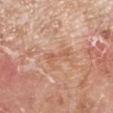No biopsy was performed on this lesion — it was imaged during a full skin examination and was not determined to be concerning. On the left lower leg. A male subject, aged 78–82. Longest diameter approximately 3.5 mm. The lesion-visualizer software estimated a lesion area of about 4 mm², an eccentricity of roughly 0.95, and two-axis asymmetry of about 0.6. The analysis additionally found a classifier nevus-likeness of about 0/100 and lesion-presence confidence of about 100/100. A close-up tile cropped from a whole-body skin photograph, about 15 mm across.